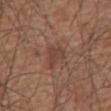Captured during whole-body skin photography for melanoma surveillance; the lesion was not biopsied. A male subject, approximately 75 years of age. A 15 mm crop from a total-body photograph taken for skin-cancer surveillance. On the back.